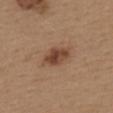Impression:
The lesion was photographed on a routine skin check and not biopsied; there is no pathology result.
Context:
The subject is a female aged around 40. The lesion is located on the back. A 15 mm close-up tile from a total-body photography series done for melanoma screening. Approximately 4 mm at its widest. The tile uses white-light illumination. The total-body-photography lesion software estimated an automated nevus-likeness rating near 90 out of 100.